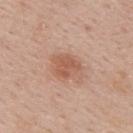follow-up: total-body-photography surveillance lesion; no biopsy | location: the mid back | diameter: ~3.5 mm (longest diameter) | subject: male, aged 53–57 | image: 15 mm crop, total-body photography.